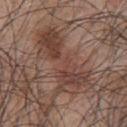biopsy status: no biopsy performed (imaged during a skin exam) | patient: male, in their mid-40s | location: the chest | acquisition: ~15 mm crop, total-body skin-cancer survey.